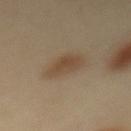Q: Patient demographics?
A: female, aged 38 to 42
Q: What kind of image is this?
A: ~15 mm tile from a whole-body skin photo
Q: Where on the body is the lesion?
A: the mid back